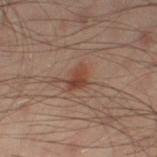Clinical impression:
Imaged during a routine full-body skin examination; the lesion was not biopsied and no histopathology is available.
Clinical summary:
The lesion is on the left leg. The patient is a male aged around 50. A close-up tile cropped from a whole-body skin photograph, about 15 mm across.